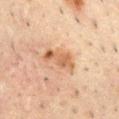biopsy status = total-body-photography surveillance lesion; no biopsy
subject = male, aged 48–52
lighting = cross-polarized
acquisition = 15 mm crop, total-body photography
diameter = ≈4.5 mm
body site = the front of the torso